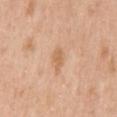Located on the mid back.
Cropped from a total-body skin-imaging series; the visible field is about 15 mm.
A female subject aged 38 to 42.